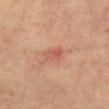Assessment: The lesion was tiled from a total-body skin photograph and was not biopsied. Background: Automated image analysis of the tile measured a mean CIELAB color near L≈46 a*≈23 b*≈27 and about 7 CIELAB-L* units darker than the surrounding skin. The software also gave border irregularity of about 2 on a 0–10 scale and peripheral color asymmetry of about 2. The tile uses cross-polarized illumination. A lesion tile, about 15 mm wide, cut from a 3D total-body photograph. The recorded lesion diameter is about 3 mm. The patient is a female approximately 40 years of age. Located on the abdomen.